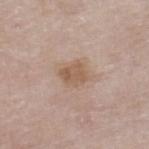workup=catalogued during a skin exam; not biopsied | patient=male, aged 78 to 82 | image=total-body-photography crop, ~15 mm field of view | illumination=white-light illumination | size=≈3 mm | automated metrics=a lesion color around L≈56 a*≈16 b*≈29 in CIELAB and roughly 9 lightness units darker than nearby skin; border irregularity of about 2.5 on a 0–10 scale, a color-variation rating of about 2.5/10, and a peripheral color-asymmetry measure near 1 | location=the right lower leg.